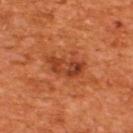| field | value |
|---|---|
| workup | no biopsy performed (imaged during a skin exam) |
| image source | ~15 mm crop, total-body skin-cancer survey |
| lesion size | about 4.5 mm |
| location | the upper back |
| automated lesion analysis | an outline eccentricity of about 0.9 (0 = round, 1 = elongated) and a shape-asymmetry score of about 0.3 (0 = symmetric); border irregularity of about 4 on a 0–10 scale, a color-variation rating of about 6.5/10, and a peripheral color-asymmetry measure near 2; lesion-presence confidence of about 100/100 |
| tile lighting | cross-polarized |
| patient | male, aged around 65 |